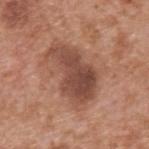Captured during whole-body skin photography for melanoma surveillance; the lesion was not biopsied. On the upper back. The patient is a male aged around 65. Cropped from a total-body skin-imaging series; the visible field is about 15 mm. The lesion's longest dimension is about 7 mm. The tile uses white-light illumination.